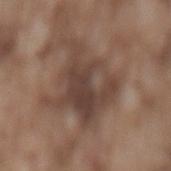Acquisition and patient details: On the lower back. An algorithmic analysis of the crop reported a mean CIELAB color near L≈42 a*≈16 b*≈23, about 10 CIELAB-L* units darker than the surrounding skin, and a normalized lesion–skin contrast near 8.5. It also reported an automated nevus-likeness rating near 0 out of 100 and a lesion-detection confidence of about 95/100. Approximately 8 mm at its widest. Cropped from a whole-body photographic skin survey; the tile spans about 15 mm. A male subject, aged approximately 75.